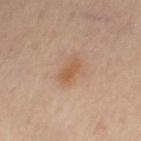The lesion was tiled from a total-body skin photograph and was not biopsied.
A female patient, aged 38 to 42.
The total-body-photography lesion software estimated a lesion–skin lightness drop of about 8 and a lesion-to-skin contrast of about 7 (normalized; higher = more distinct). The software also gave a classifier nevus-likeness of about 80/100.
The tile uses cross-polarized illumination.
About 3 mm across.
The lesion is on the right leg.
A 15 mm close-up extracted from a 3D total-body photography capture.